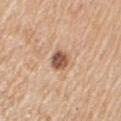  biopsy_status: not biopsied; imaged during a skin examination
  image:
    source: total-body photography crop
    field_of_view_mm: 15
  site: left upper arm
  patient:
    sex: male
    age_approx: 70